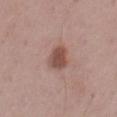Clinical impression: Recorded during total-body skin imaging; not selected for excision or biopsy. Context: The lesion's longest dimension is about 3.5 mm. Cropped from a total-body skin-imaging series; the visible field is about 15 mm. The subject is a male in their mid-70s. On the right thigh.